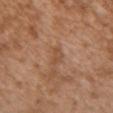Clinical impression:
Part of a total-body skin-imaging series; this lesion was reviewed on a skin check and was not flagged for biopsy.
Acquisition and patient details:
Cropped from a total-body skin-imaging series; the visible field is about 15 mm. The tile uses white-light illumination. Automated image analysis of the tile measured a footprint of about 3 mm², an outline eccentricity of about 0.8 (0 = round, 1 = elongated), and a shape-asymmetry score of about 0.35 (0 = symmetric). From the chest. A female subject, aged approximately 30.